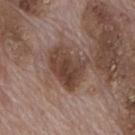<tbp_lesion>
<biopsy_status>not biopsied; imaged during a skin examination</biopsy_status>
<automated_metrics>
  <cielab_L>41</cielab_L>
  <cielab_a>18</cielab_a>
  <cielab_b>24</cielab_b>
  <vs_skin_darker_L>11.0</vs_skin_darker_L>
  <vs_skin_contrast_norm>9.0</vs_skin_contrast_norm>
  <border_irregularity_0_10>3.5</border_irregularity_0_10>
  <color_variation_0_10>5.0</color_variation_0_10>
  <nevus_likeness_0_100>10</nevus_likeness_0_100>
  <lesion_detection_confidence_0_100>100</lesion_detection_confidence_0_100>
</automated_metrics>
<lighting>white-light</lighting>
<image>
  <source>total-body photography crop</source>
  <field_of_view_mm>15</field_of_view_mm>
</image>
<patient>
  <sex>male</sex>
  <age_approx>70</age_approx>
</patient>
<site>mid back</site>
<lesion_size>
  <long_diameter_mm_approx>5.0</long_diameter_mm_approx>
</lesion_size>
</tbp_lesion>